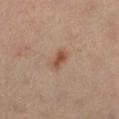No biopsy was performed on this lesion — it was imaged during a full skin examination and was not determined to be concerning.
On the left lower leg.
This image is a 15 mm lesion crop taken from a total-body photograph.
Longest diameter approximately 3 mm.
A female subject, in their 50s.
Imaged with cross-polarized lighting.